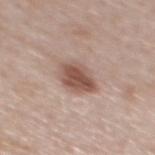Q: What is the imaging modality?
A: 15 mm crop, total-body photography
Q: What lighting was used for the tile?
A: white-light
Q: Where on the body is the lesion?
A: the back
Q: Who is the patient?
A: male, aged 38–42